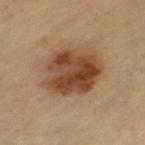biopsy status — no biopsy performed (imaged during a skin exam); patient — female, about 70 years old; lesion diameter — ≈6 mm; image source — ~15 mm crop, total-body skin-cancer survey; anatomic site — the left thigh.